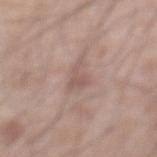• follow-up: imaged on a skin check; not biopsied
• acquisition: total-body-photography crop, ~15 mm field of view
• lesion diameter: ~3.5 mm (longest diameter)
• location: the abdomen
• subject: male, aged 68–72
• lighting: white-light illumination